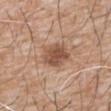Recorded during total-body skin imaging; not selected for excision or biopsy.
The subject is a male approximately 60 years of age.
Located on the chest.
Captured under white-light illumination.
Measured at roughly 4 mm in maximum diameter.
A 15 mm close-up extracted from a 3D total-body photography capture.